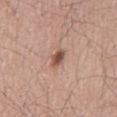Impression: This lesion was catalogued during total-body skin photography and was not selected for biopsy. Acquisition and patient details: Imaged with white-light lighting. A male patient, in their mid- to late 60s. The recorded lesion diameter is about 2.5 mm. A 15 mm close-up tile from a total-body photography series done for melanoma screening. The lesion is on the back.